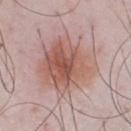Clinical impression:
Recorded during total-body skin imaging; not selected for excision or biopsy.
Acquisition and patient details:
Located on the chest. A male subject, aged around 50. A close-up tile cropped from a whole-body skin photograph, about 15 mm across.